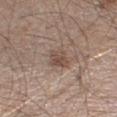Q: What is the lesion's diameter?
A: about 3 mm
Q: What is the imaging modality?
A: ~15 mm crop, total-body skin-cancer survey
Q: What are the patient's age and sex?
A: male, approximately 60 years of age
Q: Where on the body is the lesion?
A: the right lower leg
Q: Illumination type?
A: white-light